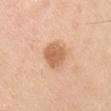The lesion was photographed on a routine skin check and not biopsied; there is no pathology result.
An algorithmic analysis of the crop reported a lesion area of about 8 mm² and an outline eccentricity of about 0.4 (0 = round, 1 = elongated). The analysis additionally found a border-irregularity rating of about 1.5/10, internal color variation of about 2.5 on a 0–10 scale, and a peripheral color-asymmetry measure near 1. The software also gave a nevus-likeness score of about 50/100 and a lesion-detection confidence of about 100/100.
From the left upper arm.
This is a white-light tile.
A lesion tile, about 15 mm wide, cut from a 3D total-body photograph.
About 3 mm across.
The patient is a male approximately 35 years of age.